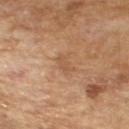Clinical impression:
Imaged during a routine full-body skin examination; the lesion was not biopsied and no histopathology is available.
Clinical summary:
Approximately 2.5 mm at its widest. A male subject, aged 63–67. Cropped from a whole-body photographic skin survey; the tile spans about 15 mm. Captured under cross-polarized illumination.